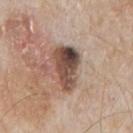Recorded during total-body skin imaging; not selected for excision or biopsy. This is a white-light tile. A male subject aged around 65. Measured at roughly 5.5 mm in maximum diameter. On the mid back. A 15 mm close-up tile from a total-body photography series done for melanoma screening.